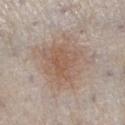This lesion was catalogued during total-body skin photography and was not selected for biopsy. Cropped from a whole-body photographic skin survey; the tile spans about 15 mm. Approximately 7 mm at its widest. A male subject, aged approximately 60. The tile uses white-light illumination. Located on the leg.